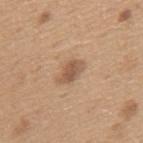The lesion was tiled from a total-body skin photograph and was not biopsied.
Approximately 3.5 mm at its widest.
Automated tile analysis of the lesion measured an automated nevus-likeness rating near 65 out of 100 and a lesion-detection confidence of about 100/100.
A 15 mm close-up tile from a total-body photography series done for melanoma screening.
Located on the mid back.
The tile uses white-light illumination.
A male subject in their mid- to late 60s.